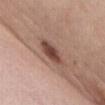notes: no biopsy performed (imaged during a skin exam)
patient: female, about 65 years old
imaging modality: 15 mm crop, total-body photography
site: the front of the torso